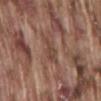Image and clinical context: A close-up tile cropped from a whole-body skin photograph, about 15 mm across. A male subject aged around 75. The lesion is located on the back. The total-body-photography lesion software estimated a lesion–skin lightness drop of about 7 and a normalized border contrast of about 5.5. And it measured a border-irregularity index near 4/10, a color-variation rating of about 2/10, and a peripheral color-asymmetry measure near 1.